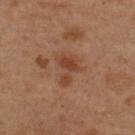biopsy status = no biopsy performed (imaged during a skin exam)
imaging modality = 15 mm crop, total-body photography
subject = male, aged 53 to 57
diameter = ~4 mm (longest diameter)
automated lesion analysis = a border-irregularity rating of about 6/10, internal color variation of about 2 on a 0–10 scale, and a peripheral color-asymmetry measure near 0.5; a lesion-detection confidence of about 100/100
body site = the upper back
illumination = cross-polarized